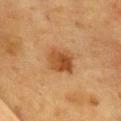<tbp_lesion>
<biopsy_status>not biopsied; imaged during a skin examination</biopsy_status>
<lighting>cross-polarized</lighting>
<patient>
  <sex>female</sex>
  <age_approx>55</age_approx>
</patient>
<automated_metrics>
  <area_mm2_approx>9.5</area_mm2_approx>
  <eccentricity>0.6</eccentricity>
  <shape_asymmetry>0.2</shape_asymmetry>
  <border_irregularity_0_10>2.0</border_irregularity_0_10>
  <color_variation_0_10>5.0</color_variation_0_10>
  <peripheral_color_asymmetry>2.0</peripheral_color_asymmetry>
</automated_metrics>
<lesion_size>
  <long_diameter_mm_approx>3.5</long_diameter_mm_approx>
</lesion_size>
<image>
  <source>total-body photography crop</source>
  <field_of_view_mm>15</field_of_view_mm>
</image>
<site>chest</site>
</tbp_lesion>